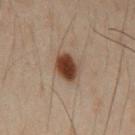Notes:
- notes · no biopsy performed (imaged during a skin exam)
- diameter · about 4 mm
- image-analysis metrics · a footprint of about 7 mm² and a symmetry-axis asymmetry near 0.2; an average lesion color of about L≈30 a*≈15 b*≈22 (CIELAB), a lesion–skin lightness drop of about 14, and a normalized border contrast of about 13; a border-irregularity rating of about 2/10, a within-lesion color-variation index near 3/10, and a peripheral color-asymmetry measure near 1; an automated nevus-likeness rating near 100 out of 100 and a detector confidence of about 100 out of 100 that the crop contains a lesion
- body site · the chest
- subject · male, about 50 years old
- tile lighting · cross-polarized
- acquisition · 15 mm crop, total-body photography